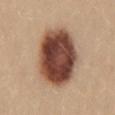Clinical impression:
Recorded during total-body skin imaging; not selected for excision or biopsy.
Image and clinical context:
The subject is a female aged around 25. An algorithmic analysis of the crop reported an automated nevus-likeness rating near 100 out of 100 and lesion-presence confidence of about 100/100. Imaged with white-light lighting. This image is a 15 mm lesion crop taken from a total-body photograph. On the mid back. Approximately 8 mm at its widest.